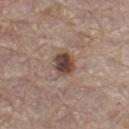Cropped from a whole-body photographic skin survey; the tile spans about 15 mm. The patient is a male aged 63–67. From the left thigh. Imaged with white-light lighting.